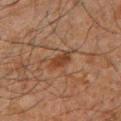The lesion was photographed on a routine skin check and not biopsied; there is no pathology result. On the front of the torso. The recorded lesion diameter is about 3.5 mm. The subject is a male aged 58 to 62. The lesion-visualizer software estimated an outline eccentricity of about 0.9 (0 = round, 1 = elongated) and a symmetry-axis asymmetry near 0.35. It also reported a within-lesion color-variation index near 2.5/10 and a peripheral color-asymmetry measure near 1. And it measured a classifier nevus-likeness of about 45/100. A lesion tile, about 15 mm wide, cut from a 3D total-body photograph. Captured under cross-polarized illumination.